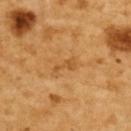{"biopsy_status": "not biopsied; imaged during a skin examination", "automated_metrics": {"cielab_L": 54, "cielab_a": 23, "cielab_b": 45, "vs_skin_darker_L": 8.0, "vs_skin_contrast_norm": 5.5, "color_variation_0_10": 0.0, "peripheral_color_asymmetry": 0.0, "nevus_likeness_0_100": 0, "lesion_detection_confidence_0_100": 100}, "site": "upper back", "image": {"source": "total-body photography crop", "field_of_view_mm": 15}, "lighting": "cross-polarized", "patient": {"sex": "female", "age_approx": 55}}